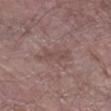Q: Was this lesion biopsied?
A: imaged on a skin check; not biopsied
Q: How was this image acquired?
A: total-body-photography crop, ~15 mm field of view
Q: What is the lesion's diameter?
A: about 4.5 mm
Q: What did automated image analysis measure?
A: a lesion area of about 6.5 mm², an outline eccentricity of about 0.9 (0 = round, 1 = elongated), and a shape-asymmetry score of about 0.35 (0 = symmetric); an average lesion color of about L≈48 a*≈17 b*≈20 (CIELAB) and about 6 CIELAB-L* units darker than the surrounding skin; a classifier nevus-likeness of about 0/100 and lesion-presence confidence of about 95/100
Q: Who is the patient?
A: male, about 80 years old
Q: What is the anatomic site?
A: the leg